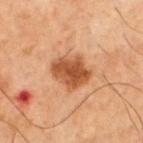Part of a total-body skin-imaging series; this lesion was reviewed on a skin check and was not flagged for biopsy. This is a cross-polarized tile. A male subject, in their 70s. A region of skin cropped from a whole-body photographic capture, roughly 15 mm wide. The lesion is located on the chest. Measured at roughly 4.5 mm in maximum diameter.